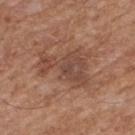Part of a total-body skin-imaging series; this lesion was reviewed on a skin check and was not flagged for biopsy. The patient is a male in their mid-60s. On the back. A lesion tile, about 15 mm wide, cut from a 3D total-body photograph.